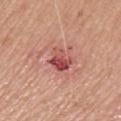{
  "biopsy_status": "not biopsied; imaged during a skin examination",
  "patient": {
    "sex": "male",
    "age_approx": 65
  },
  "image": {
    "source": "total-body photography crop",
    "field_of_view_mm": 15
  },
  "site": "head or neck",
  "lighting": "white-light"
}